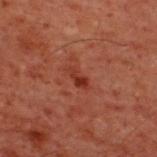Cropped from a total-body skin-imaging series; the visible field is about 15 mm.
A male patient aged approximately 60.
The total-body-photography lesion software estimated a footprint of about 3 mm², a shape eccentricity near 0.9, and a shape-asymmetry score of about 0.3 (0 = symmetric). The analysis additionally found an average lesion color of about L≈26 a*≈25 b*≈26 (CIELAB) and about 7 CIELAB-L* units darker than the surrounding skin. And it measured an automated nevus-likeness rating near 5 out of 100 and a lesion-detection confidence of about 100/100.
Imaged with cross-polarized lighting.
The lesion is located on the upper back.